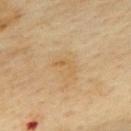workup: imaged on a skin check; not biopsied | patient: female, aged 58–62 | image source: ~15 mm tile from a whole-body skin photo | lighting: cross-polarized | body site: the upper back.